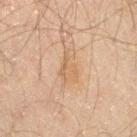Findings:
* workup — no biopsy performed (imaged during a skin exam)
* site — the right thigh
* lesion diameter — about 4 mm
* imaging modality — ~15 mm crop, total-body skin-cancer survey
* subject — male, aged approximately 60